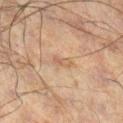Clinical impression:
Recorded during total-body skin imaging; not selected for excision or biopsy.
Image and clinical context:
Automated tile analysis of the lesion measured a lesion color around L≈45 a*≈15 b*≈25 in CIELAB, roughly 6 lightness units darker than nearby skin, and a lesion-to-skin contrast of about 5 (normalized; higher = more distinct). And it measured a border-irregularity rating of about 3.5/10, a color-variation rating of about 0/10, and a peripheral color-asymmetry measure near 0. And it measured lesion-presence confidence of about 95/100. Imaged with cross-polarized lighting. A male subject about 60 years old. This image is a 15 mm lesion crop taken from a total-body photograph. The lesion is on the left leg. The recorded lesion diameter is about 3 mm.